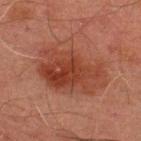On the upper back. The recorded lesion diameter is about 8 mm. Imaged with cross-polarized lighting. This image is a 15 mm lesion crop taken from a total-body photograph. The lesion-visualizer software estimated a mean CIELAB color near L≈35 a*≈23 b*≈26, roughly 8 lightness units darker than nearby skin, and a normalized lesion–skin contrast near 7. And it measured a lesion-detection confidence of about 100/100. A male patient in their mid-40s.